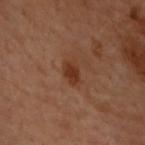notes = catalogued during a skin exam; not biopsied
patient = female, in their 60s
location = the back
lesion size = about 3 mm
acquisition = 15 mm crop, total-body photography
tile lighting = cross-polarized
image-analysis metrics = a nevus-likeness score of about 70/100 and a detector confidence of about 100 out of 100 that the crop contains a lesion